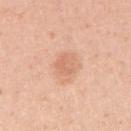Imaged during a routine full-body skin examination; the lesion was not biopsied and no histopathology is available.
Approximately 2.5 mm at its widest.
A female patient, aged around 30.
Automated image analysis of the tile measured a lesion color around L≈67 a*≈24 b*≈33 in CIELAB and a lesion–skin lightness drop of about 8. It also reported a border-irregularity rating of about 2.5/10, a color-variation rating of about 2/10, and peripheral color asymmetry of about 0.5. The software also gave an automated nevus-likeness rating near 15 out of 100.
On the right upper arm.
A 15 mm close-up tile from a total-body photography series done for melanoma screening.
The tile uses white-light illumination.